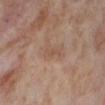The lesion was photographed on a routine skin check and not biopsied; there is no pathology result. A 15 mm crop from a total-body photograph taken for skin-cancer surveillance. From the right lower leg. The tile uses cross-polarized illumination. A female patient, aged approximately 55. Longest diameter approximately 2.5 mm.